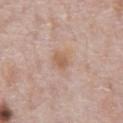No biopsy was performed on this lesion — it was imaged during a full skin examination and was not determined to be concerning.
Approximately 4 mm at its widest.
Cropped from a total-body skin-imaging series; the visible field is about 15 mm.
A male patient about 70 years old.
On the chest.